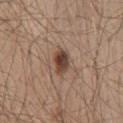notes: imaged on a skin check; not biopsied
patient: male, aged around 45
image: 15 mm crop, total-body photography
site: the back
lighting: white-light illumination
image-analysis metrics: two-axis asymmetry of about 0.2
size: ≈3.5 mm